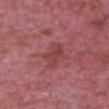This lesion was catalogued during total-body skin photography and was not selected for biopsy.
The patient is a male aged approximately 65.
Captured under white-light illumination.
The lesion is on the chest.
A region of skin cropped from a whole-body photographic capture, roughly 15 mm wide.
Longest diameter approximately 3.5 mm.